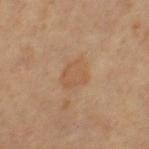- workup: catalogued during a skin exam; not biopsied
- acquisition: ~15 mm tile from a whole-body skin photo
- size: ≈3 mm
- site: the leg
- image-analysis metrics: an area of roughly 7 mm², an outline eccentricity of about 0.5 (0 = round, 1 = elongated), and a symmetry-axis asymmetry near 0.2; an average lesion color of about L≈53 a*≈19 b*≈33 (CIELAB), a lesion–skin lightness drop of about 6, and a normalized lesion–skin contrast near 4.5; a classifier nevus-likeness of about 5/100 and a lesion-detection confidence of about 100/100
- lighting: cross-polarized illumination
- subject: female, in their mid- to late 60s